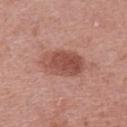| feature | finding |
|---|---|
| notes | catalogued during a skin exam; not biopsied |
| lesion diameter | about 5.5 mm |
| subject | female, roughly 40 years of age |
| image | 15 mm crop, total-body photography |
| lighting | white-light |
| location | the upper back |
| image-analysis metrics | a lesion area of about 15 mm², an eccentricity of roughly 0.8, and two-axis asymmetry of about 0.1 |